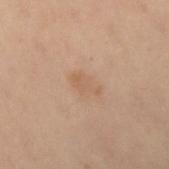{
  "biopsy_status": "not biopsied; imaged during a skin examination"
}